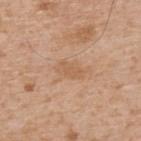The lesion was photographed on a routine skin check and not biopsied; there is no pathology result. The subject is a male roughly 65 years of age. Approximately 3 mm at its widest. The lesion is on the upper back. This image is a 15 mm lesion crop taken from a total-body photograph. This is a white-light tile.